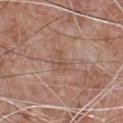Findings:
* workup — total-body-photography surveillance lesion; no biopsy
* diameter — about 2.5 mm
* illumination — white-light illumination
* anatomic site — the chest
* subject — male, approximately 65 years of age
* image — 15 mm crop, total-body photography